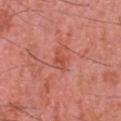| field | value |
|---|---|
| imaging modality | ~15 mm tile from a whole-body skin photo |
| subject | male, roughly 45 years of age |
| body site | the head or neck |
| illumination | white-light |
| lesion diameter | ≈2.5 mm |